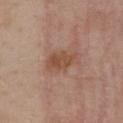From the chest. This image is a 15 mm lesion crop taken from a total-body photograph. Captured under white-light illumination. Automated tile analysis of the lesion measured border irregularity of about 3 on a 0–10 scale, a color-variation rating of about 3/10, and peripheral color asymmetry of about 1. The analysis additionally found an automated nevus-likeness rating near 40 out of 100 and lesion-presence confidence of about 100/100. The lesion's longest dimension is about 4.5 mm. The patient is a female aged around 55.